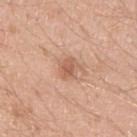<tbp_lesion>
  <image>
    <source>total-body photography crop</source>
    <field_of_view_mm>15</field_of_view_mm>
  </image>
  <site>left upper arm</site>
  <automated_metrics>
    <border_irregularity_0_10>1.5</border_irregularity_0_10>
    <color_variation_0_10>2.5</color_variation_0_10>
    <peripheral_color_asymmetry>1.0</peripheral_color_asymmetry>
  </automated_metrics>
  <lighting>white-light</lighting>
  <lesion_size>
    <long_diameter_mm_approx>2.5</long_diameter_mm_approx>
  </lesion_size>
  <patient>
    <sex>male</sex>
    <age_approx>20</age_approx>
  </patient>
</tbp_lesion>